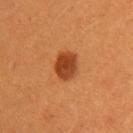<lesion>
<patient>
  <sex>female</sex>
  <age_approx>30</age_approx>
</patient>
<image>
  <source>total-body photography crop</source>
  <field_of_view_mm>15</field_of_view_mm>
</image>
<lighting>cross-polarized</lighting>
<site>left upper arm</site>
</lesion>